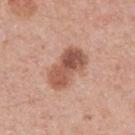Findings:
• biopsy status — total-body-photography surveillance lesion; no biopsy
• subject — female, aged 33–37
• size — ≈5.5 mm
• body site — the left forearm
• lighting — white-light
• acquisition — 15 mm crop, total-body photography
• automated metrics — border irregularity of about 2.5 on a 0–10 scale and internal color variation of about 7 on a 0–10 scale